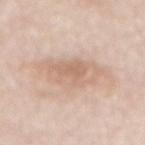Recorded during total-body skin imaging; not selected for excision or biopsy. The subject is a female roughly 65 years of age. A lesion tile, about 15 mm wide, cut from a 3D total-body photograph. From the mid back. The tile uses white-light illumination.